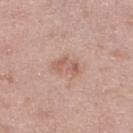Clinical impression: Imaged during a routine full-body skin examination; the lesion was not biopsied and no histopathology is available. Clinical summary: About 3.5 mm across. The tile uses white-light illumination. Located on the right lower leg. A male subject aged around 50. Cropped from a total-body skin-imaging series; the visible field is about 15 mm. An algorithmic analysis of the crop reported a lesion area of about 4.5 mm² and a shape-asymmetry score of about 0.4 (0 = symmetric). The analysis additionally found a mean CIELAB color near L≈59 a*≈22 b*≈27, roughly 9 lightness units darker than nearby skin, and a lesion-to-skin contrast of about 6 (normalized; higher = more distinct).